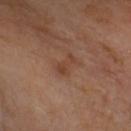Part of a total-body skin-imaging series; this lesion was reviewed on a skin check and was not flagged for biopsy.
The lesion is located on the head or neck.
This is a cross-polarized tile.
A female subject aged 68–72.
Cropped from a whole-body photographic skin survey; the tile spans about 15 mm.
The recorded lesion diameter is about 3 mm.